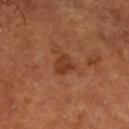Part of a total-body skin-imaging series; this lesion was reviewed on a skin check and was not flagged for biopsy.
A lesion tile, about 15 mm wide, cut from a 3D total-body photograph.
Measured at roughly 2.5 mm in maximum diameter.
A male patient in their mid-60s.
The lesion is located on the right lower leg.